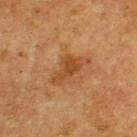Imaged during a routine full-body skin examination; the lesion was not biopsied and no histopathology is available. A male patient roughly 75 years of age. This image is a 15 mm lesion crop taken from a total-body photograph. The lesion is on the upper back.